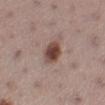The lesion was tiled from a total-body skin photograph and was not biopsied.
A female subject, in their mid- to late 50s.
The lesion is located on the left lower leg.
This image is a 15 mm lesion crop taken from a total-body photograph.